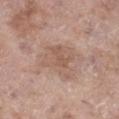Q: Is there a histopathology result?
A: catalogued during a skin exam; not biopsied
Q: Lesion location?
A: the leg
Q: What are the patient's age and sex?
A: female, in their mid- to late 70s
Q: What is the imaging modality?
A: total-body-photography crop, ~15 mm field of view